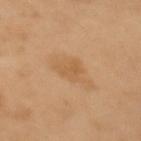| key | value |
|---|---|
| follow-up | total-body-photography surveillance lesion; no biopsy |
| subject | female, approximately 55 years of age |
| lesion diameter | about 3 mm |
| automated metrics | a normalized border contrast of about 5; a classifier nevus-likeness of about 0/100 and a detector confidence of about 100 out of 100 that the crop contains a lesion |
| imaging modality | total-body-photography crop, ~15 mm field of view |
| body site | the arm |